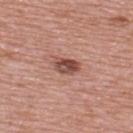The lesion was photographed on a routine skin check and not biopsied; there is no pathology result. Located on the upper back. A male subject approximately 70 years of age. Cropped from a total-body skin-imaging series; the visible field is about 15 mm.